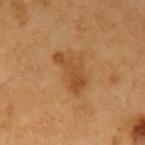The lesion was tiled from a total-body skin photograph and was not biopsied.
The lesion is on the left upper arm.
About 5 mm across.
Cropped from a total-body skin-imaging series; the visible field is about 15 mm.
A male patient aged 58 to 62.